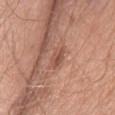Image and clinical context: A female subject approximately 65 years of age. A 15 mm crop from a total-body photograph taken for skin-cancer surveillance. The lesion is on the head or neck.